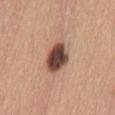Case summary:
– follow-up — total-body-photography surveillance lesion; no biopsy
– lesion size — about 4 mm
– illumination — white-light
– patient — female, aged 28–32
– image — total-body-photography crop, ~15 mm field of view
– anatomic site — the front of the torso